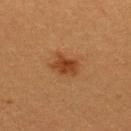| feature | finding |
|---|---|
| workup | imaged on a skin check; not biopsied |
| lesion diameter | about 3 mm |
| image | ~15 mm tile from a whole-body skin photo |
| subject | female, roughly 20 years of age |
| automated metrics | a within-lesion color-variation index near 2.5/10 and radial color variation of about 0.5 |
| anatomic site | the upper back |